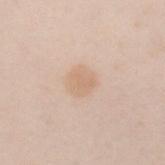biopsy status = catalogued during a skin exam; not biopsied | subject = female, roughly 20 years of age | image source = ~15 mm tile from a whole-body skin photo | lighting = white-light illumination | lesion diameter = ~2.5 mm (longest diameter) | body site = the left forearm | automated metrics = a mean CIELAB color near L≈68 a*≈17 b*≈31, a lesion–skin lightness drop of about 7, and a normalized border contrast of about 5.5; a detector confidence of about 100 out of 100 that the crop contains a lesion.